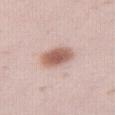Recorded during total-body skin imaging; not selected for excision or biopsy. A female patient in their mid- to late 20s. About 4 mm across. Located on the right thigh. A close-up tile cropped from a whole-body skin photograph, about 15 mm across.